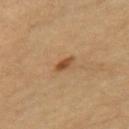<tbp_lesion>
<biopsy_status>not biopsied; imaged during a skin examination</biopsy_status>
<patient>
  <sex>female</sex>
  <age_approx>70</age_approx>
</patient>
<image>
  <source>total-body photography crop</source>
  <field_of_view_mm>15</field_of_view_mm>
</image>
<site>right thigh</site>
</tbp_lesion>